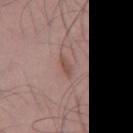Q: Is there a histopathology result?
A: total-body-photography surveillance lesion; no biopsy
Q: What are the patient's age and sex?
A: male, aged approximately 45
Q: What is the imaging modality?
A: ~15 mm tile from a whole-body skin photo
Q: Illumination type?
A: white-light
Q: Lesion size?
A: about 3 mm
Q: What is the anatomic site?
A: the mid back